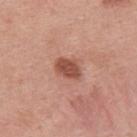Impression:
This lesion was catalogued during total-body skin photography and was not selected for biopsy.
Image and clinical context:
Measured at roughly 3 mm in maximum diameter. Imaged with white-light lighting. A lesion tile, about 15 mm wide, cut from a 3D total-body photograph. On the back. A male subject in their mid-20s.